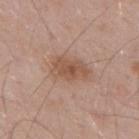Findings:
- follow-up — imaged on a skin check; not biopsied
- anatomic site — the mid back
- lesion diameter — ≈4 mm
- TBP lesion metrics — an automated nevus-likeness rating near 25 out of 100 and lesion-presence confidence of about 100/100
- lighting — white-light
- subject — male, about 55 years old
- image — 15 mm crop, total-body photography